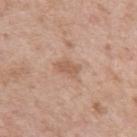{
  "biopsy_status": "not biopsied; imaged during a skin examination",
  "lesion_size": {
    "long_diameter_mm_approx": 2.5
  },
  "patient": {
    "sex": "male",
    "age_approx": 50
  },
  "site": "left upper arm",
  "lighting": "white-light",
  "automated_metrics": {
    "cielab_L": 58,
    "cielab_a": 20,
    "cielab_b": 30,
    "vs_skin_darker_L": 9.0,
    "vs_skin_contrast_norm": 6.0,
    "border_irregularity_0_10": 3.0,
    "color_variation_0_10": 1.0,
    "peripheral_color_asymmetry": 0.5,
    "nevus_likeness_0_100": 0,
    "lesion_detection_confidence_0_100": 100
  },
  "image": {
    "source": "total-body photography crop",
    "field_of_view_mm": 15
  }
}